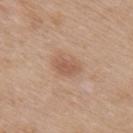biopsy_status: not biopsied; imaged during a skin examination
image:
  source: total-body photography crop
  field_of_view_mm: 15
patient:
  sex: female
  age_approx: 60
site: upper back